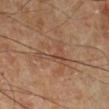notes = catalogued during a skin exam; not biopsied
lighting = cross-polarized
lesion size = ≈5 mm
patient = male, approximately 70 years of age
site = the right lower leg
image source = ~15 mm tile from a whole-body skin photo
image-analysis metrics = an eccentricity of roughly 0.9 and a symmetry-axis asymmetry near 0.75